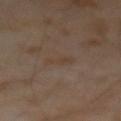Image and clinical context:
Automated image analysis of the tile measured an eccentricity of roughly 0.85. The analysis additionally found a border-irregularity rating of about 3.5/10, internal color variation of about 1 on a 0–10 scale, and radial color variation of about 0. The software also gave a nevus-likeness score of about 0/100. About 2.5 mm across. A region of skin cropped from a whole-body photographic capture, roughly 15 mm wide. A male patient, in their mid-60s.